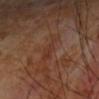Impression:
This lesion was catalogued during total-body skin photography and was not selected for biopsy.
Clinical summary:
Cropped from a whole-body photographic skin survey; the tile spans about 15 mm. On the left forearm. A male patient, in their 70s.